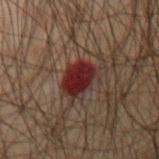Clinical impression:
No biopsy was performed on this lesion — it was imaged during a full skin examination and was not determined to be concerning.
Clinical summary:
A male patient aged 48 to 52. A lesion tile, about 15 mm wide, cut from a 3D total-body photograph. Located on the arm.